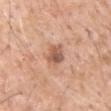Clinical impression:
The lesion was tiled from a total-body skin photograph and was not biopsied.
Context:
An algorithmic analysis of the crop reported a footprint of about 5.5 mm² and two-axis asymmetry of about 0.25. It also reported a border-irregularity index near 2.5/10, a color-variation rating of about 6/10, and a peripheral color-asymmetry measure near 2. The analysis additionally found a classifier nevus-likeness of about 25/100. The tile uses white-light illumination. Approximately 3 mm at its widest. A male subject, aged 58 to 62. On the arm. A 15 mm crop from a total-body photograph taken for skin-cancer surveillance.